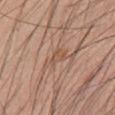Assessment:
Part of a total-body skin-imaging series; this lesion was reviewed on a skin check and was not flagged for biopsy.
Clinical summary:
This is a white-light tile. The patient is a male aged approximately 60. Cropped from a whole-body photographic skin survey; the tile spans about 15 mm. On the abdomen.